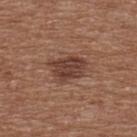- subject: female, aged 73 to 77
- location: the upper back
- image source: ~15 mm crop, total-body skin-cancer survey